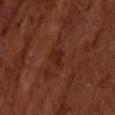notes = catalogued during a skin exam; not biopsied | image = total-body-photography crop, ~15 mm field of view | anatomic site = the upper back | lesion size = ~2.5 mm (longest diameter) | subject = male, aged approximately 70.